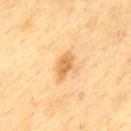{"patient": {"sex": "male", "age_approx": 70}, "site": "back", "lighting": "cross-polarized", "automated_metrics": {"cielab_L": 57, "cielab_a": 19, "cielab_b": 39, "vs_skin_darker_L": 10.0, "vs_skin_contrast_norm": 7.5, "border_irregularity_0_10": 2.5, "color_variation_0_10": 2.5, "peripheral_color_asymmetry": 0.5, "nevus_likeness_0_100": 65}, "image": {"source": "total-body photography crop", "field_of_view_mm": 15}, "lesion_size": {"long_diameter_mm_approx": 3.5}}